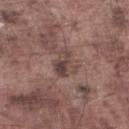Notes:
* notes · imaged on a skin check; not biopsied
* automated lesion analysis · a nevus-likeness score of about 0/100 and lesion-presence confidence of about 85/100
* lesion size · about 3.5 mm
* anatomic site · the left lower leg
* subject · male, roughly 75 years of age
* imaging modality · ~15 mm tile from a whole-body skin photo